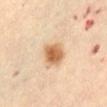This lesion was catalogued during total-body skin photography and was not selected for biopsy. The lesion is on the abdomen. About 5 mm across. The tile uses cross-polarized illumination. This image is a 15 mm lesion crop taken from a total-body photograph. A female subject, aged approximately 65.